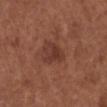Clinical impression:
Captured during whole-body skin photography for melanoma surveillance; the lesion was not biopsied.
Context:
Located on the right lower leg. A 15 mm close-up extracted from a 3D total-body photography capture. The patient is a female approximately 55 years of age. This is a white-light tile.